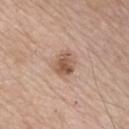Impression:
Imaged during a routine full-body skin examination; the lesion was not biopsied and no histopathology is available.
Background:
Located on the front of the torso. A male patient, in their mid-60s. Cropped from a whole-body photographic skin survey; the tile spans about 15 mm.